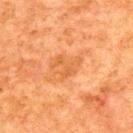Imaged during a routine full-body skin examination; the lesion was not biopsied and no histopathology is available.
A male patient aged around 80.
From the upper back.
A lesion tile, about 15 mm wide, cut from a 3D total-body photograph.